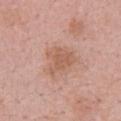Q: Is there a histopathology result?
A: catalogued during a skin exam; not biopsied
Q: What did automated image analysis measure?
A: a footprint of about 9 mm², a shape eccentricity near 0.45, and a shape-asymmetry score of about 0.45 (0 = symmetric)
Q: Patient demographics?
A: female, aged around 70
Q: Lesion size?
A: ≈4 mm
Q: What is the imaging modality?
A: ~15 mm tile from a whole-body skin photo
Q: Lesion location?
A: the chest
Q: What lighting was used for the tile?
A: white-light